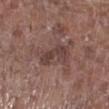Clinical impression:
Part of a total-body skin-imaging series; this lesion was reviewed on a skin check and was not flagged for biopsy.
Context:
A 15 mm close-up tile from a total-body photography series done for melanoma screening. An algorithmic analysis of the crop reported an average lesion color of about L≈40 a*≈18 b*≈20 (CIELAB), about 9 CIELAB-L* units darker than the surrounding skin, and a normalized lesion–skin contrast near 7. And it measured a classifier nevus-likeness of about 0/100 and a lesion-detection confidence of about 100/100. A male subject, aged around 55. Located on the leg. The recorded lesion diameter is about 4.5 mm.